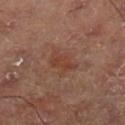Impression:
This lesion was catalogued during total-body skin photography and was not selected for biopsy.
Image and clinical context:
A region of skin cropped from a whole-body photographic capture, roughly 15 mm wide. Imaged with cross-polarized lighting. The lesion is located on the leg. Approximately 3.5 mm at its widest.